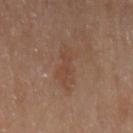Q: Is there a histopathology result?
A: imaged on a skin check; not biopsied
Q: Patient demographics?
A: female, aged around 65
Q: Lesion location?
A: the left upper arm
Q: Illumination type?
A: cross-polarized
Q: How was this image acquired?
A: ~15 mm crop, total-body skin-cancer survey
Q: How large is the lesion?
A: ~5 mm (longest diameter)
Q: Automated lesion metrics?
A: an area of roughly 9 mm² and an eccentricity of roughly 0.9